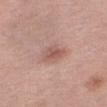follow-up: catalogued during a skin exam; not biopsied | subject: female, aged 38–42 | anatomic site: the right thigh | illumination: white-light | acquisition: ~15 mm tile from a whole-body skin photo | automated lesion analysis: a lesion area of about 6 mm², an outline eccentricity of about 0.65 (0 = round, 1 = elongated), and a symmetry-axis asymmetry near 0.25.